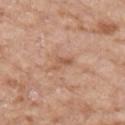Notes:
– follow-up: total-body-photography surveillance lesion; no biopsy
– size: ~3 mm (longest diameter)
– image source: 15 mm crop, total-body photography
– anatomic site: the right upper arm
– automated lesion analysis: a footprint of about 3 mm² and a symmetry-axis asymmetry near 0.5; a lesion color around L≈57 a*≈21 b*≈32 in CIELAB and a lesion-to-skin contrast of about 5.5 (normalized; higher = more distinct); border irregularity of about 5 on a 0–10 scale, a color-variation rating of about 0/10, and peripheral color asymmetry of about 0; a classifier nevus-likeness of about 0/100 and a detector confidence of about 100 out of 100 that the crop contains a lesion
– tile lighting: white-light illumination
– subject: female, aged 73 to 77